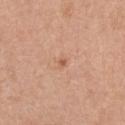Clinical impression:
Imaged during a routine full-body skin examination; the lesion was not biopsied and no histopathology is available.
Background:
Approximately 1 mm at its widest. The lesion is on the chest. The subject is a female approximately 30 years of age. A 15 mm close-up tile from a total-body photography series done for melanoma screening.